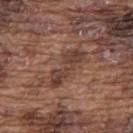Recorded during total-body skin imaging; not selected for excision or biopsy.
This is a white-light tile.
A lesion tile, about 15 mm wide, cut from a 3D total-body photograph.
About 5.5 mm across.
Located on the upper back.
An algorithmic analysis of the crop reported an average lesion color of about L≈40 a*≈19 b*≈24 (CIELAB) and a normalized lesion–skin contrast near 8. And it measured a border-irregularity index near 7.5/10, a within-lesion color-variation index near 3.5/10, and a peripheral color-asymmetry measure near 1.
A male patient, roughly 75 years of age.